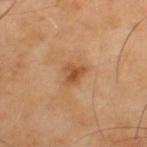Clinical impression: Recorded during total-body skin imaging; not selected for excision or biopsy. Context: A 15 mm close-up extracted from a 3D total-body photography capture. A male subject, approximately 40 years of age. The lesion is on the upper back. About 2.5 mm across.